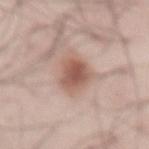<case>
  <patient>
    <sex>male</sex>
    <age_approx>55</age_approx>
  </patient>
  <lighting>white-light</lighting>
  <image>
    <source>total-body photography crop</source>
    <field_of_view_mm>15</field_of_view_mm>
  </image>
  <site>abdomen</site>
</case>